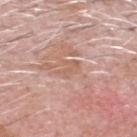Q: Is there a histopathology result?
A: catalogued during a skin exam; not biopsied
Q: What are the patient's age and sex?
A: male, in their 70s
Q: What is the imaging modality?
A: ~15 mm tile from a whole-body skin photo
Q: Lesion location?
A: the head or neck